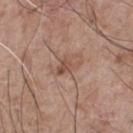follow-up=no biopsy performed (imaged during a skin exam) | lesion diameter=~3.5 mm (longest diameter) | image source=15 mm crop, total-body photography | anatomic site=the chest | patient=male, aged 73–77.